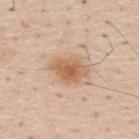{"biopsy_status": "not biopsied; imaged during a skin examination"}